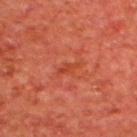workup: no biopsy performed (imaged during a skin exam) | subject: male, aged 63 to 67 | tile lighting: cross-polarized illumination | acquisition: ~15 mm crop, total-body skin-cancer survey | image-analysis metrics: an area of roughly 2.5 mm², an outline eccentricity of about 0.9 (0 = round, 1 = elongated), and a shape-asymmetry score of about 0.35 (0 = symmetric); a lesion color around L≈41 a*≈33 b*≈35 in CIELAB and about 6 CIELAB-L* units darker than the surrounding skin; border irregularity of about 4 on a 0–10 scale and internal color variation of about 0 on a 0–10 scale; a classifier nevus-likeness of about 0/100 and lesion-presence confidence of about 100/100 | body site: the upper back.